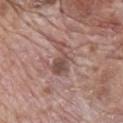Case summary:
* notes — no biopsy performed (imaged during a skin exam)
* anatomic site — the mid back
* image — 15 mm crop, total-body photography
* patient — male, roughly 70 years of age